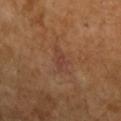Findings:
• notes: no biopsy performed (imaged during a skin exam)
• body site: the arm
• lighting: cross-polarized illumination
• automated metrics: a lesion area of about 5 mm² and an eccentricity of roughly 0.85; a border-irregularity rating of about 4/10, a color-variation rating of about 2.5/10, and radial color variation of about 1; a nevus-likeness score of about 0/100 and a lesion-detection confidence of about 100/100
• acquisition: 15 mm crop, total-body photography
• subject: female, in their 70s